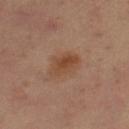Imaged during a routine full-body skin examination; the lesion was not biopsied and no histopathology is available. A female subject aged 38–42. The lesion is located on the right lower leg. Cropped from a whole-body photographic skin survey; the tile spans about 15 mm. Longest diameter approximately 4 mm. This is a cross-polarized tile. Automated image analysis of the tile measured a footprint of about 8.5 mm², a shape eccentricity near 0.75, and a symmetry-axis asymmetry near 0.25. It also reported a mean CIELAB color near L≈45 a*≈20 b*≈31. The analysis additionally found a border-irregularity index near 2.5/10, internal color variation of about 4 on a 0–10 scale, and a peripheral color-asymmetry measure near 1.5. It also reported an automated nevus-likeness rating near 90 out of 100.